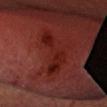biopsy status — imaged on a skin check; not biopsied | patient — male, roughly 60 years of age | site — the head or neck | image — ~15 mm crop, total-body skin-cancer survey.